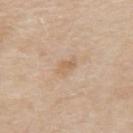Imaged during a routine full-body skin examination; the lesion was not biopsied and no histopathology is available. This image is a 15 mm lesion crop taken from a total-body photograph. The lesion's longest dimension is about 2.5 mm. The lesion is on the upper back. A female subject, aged 68 to 72. Captured under white-light illumination. An algorithmic analysis of the crop reported an average lesion color of about L≈63 a*≈16 b*≈33 (CIELAB), about 7 CIELAB-L* units darker than the surrounding skin, and a lesion-to-skin contrast of about 5 (normalized; higher = more distinct). It also reported an automated nevus-likeness rating near 0 out of 100 and a detector confidence of about 100 out of 100 that the crop contains a lesion.